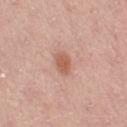notes = total-body-photography surveillance lesion; no biopsy | illumination = white-light | automated lesion analysis = a lesion–skin lightness drop of about 10; a classifier nevus-likeness of about 90/100 | image source = ~15 mm tile from a whole-body skin photo | subject = female, approximately 50 years of age | anatomic site = the right thigh.